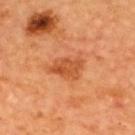Impression: Captured during whole-body skin photography for melanoma surveillance; the lesion was not biopsied. Clinical summary: A male subject approximately 65 years of age. A 15 mm crop from a total-body photograph taken for skin-cancer surveillance. This is a cross-polarized tile. From the upper back.